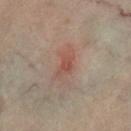Recorded during total-body skin imaging; not selected for excision or biopsy. On the leg. The tile uses cross-polarized illumination. A female subject roughly 60 years of age. The recorded lesion diameter is about 3.5 mm. A roughly 15 mm field-of-view crop from a total-body skin photograph.